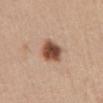Assessment:
Captured during whole-body skin photography for melanoma surveillance; the lesion was not biopsied.
Acquisition and patient details:
Measured at roughly 3.5 mm in maximum diameter. This image is a 15 mm lesion crop taken from a total-body photograph. This is a white-light tile. A female patient in their mid-40s. Automated image analysis of the tile measured an eccentricity of roughly 0.55 and a symmetry-axis asymmetry near 0.2. And it measured border irregularity of about 2 on a 0–10 scale and peripheral color asymmetry of about 1.5. The software also gave a classifier nevus-likeness of about 100/100 and a lesion-detection confidence of about 100/100. On the abdomen.